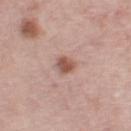This lesion was catalogued during total-body skin photography and was not selected for biopsy.
A 15 mm crop from a total-body photograph taken for skin-cancer surveillance.
From the left thigh.
A female subject aged 63 to 67.